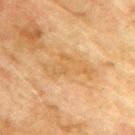Impression:
No biopsy was performed on this lesion — it was imaged during a full skin examination and was not determined to be concerning.
Acquisition and patient details:
Captured under cross-polarized illumination. A male subject in their mid-70s. This image is a 15 mm lesion crop taken from a total-body photograph. Longest diameter approximately 6.5 mm. The lesion is located on the upper back.